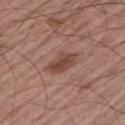No biopsy was performed on this lesion — it was imaged during a full skin examination and was not determined to be concerning.
An algorithmic analysis of the crop reported a mean CIELAB color near L≈45 a*≈21 b*≈26 and roughly 10 lightness units darker than nearby skin.
The patient is a male roughly 65 years of age.
A 15 mm close-up extracted from a 3D total-body photography capture.
Imaged with white-light lighting.
Located on the left upper arm.
Longest diameter approximately 3.5 mm.